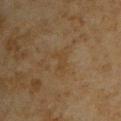Imaged during a routine full-body skin examination; the lesion was not biopsied and no histopathology is available.
Located on the upper back.
About 3 mm across.
The patient is a male in their mid-40s.
Automated tile analysis of the lesion measured a footprint of about 3.5 mm², an eccentricity of roughly 0.85, and a shape-asymmetry score of about 0.55 (0 = symmetric). The analysis additionally found a border-irregularity index near 6/10, a color-variation rating of about 1/10, and radial color variation of about 0.
A 15 mm crop from a total-body photograph taken for skin-cancer surveillance.
This is a cross-polarized tile.